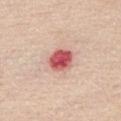<case>
  <biopsy_status>not biopsied; imaged during a skin examination</biopsy_status>
  <automated_metrics>
    <vs_skin_darker_L>18.0</vs_skin_darker_L>
    <vs_skin_contrast_norm>11.0</vs_skin_contrast_norm>
    <border_irregularity_0_10>1.5</border_irregularity_0_10>
    <color_variation_0_10>5.0</color_variation_0_10>
    <peripheral_color_asymmetry>1.5</peripheral_color_asymmetry>
  </automated_metrics>
  <lighting>white-light</lighting>
  <patient>
    <sex>male</sex>
    <age_approx>80</age_approx>
  </patient>
  <lesion_size>
    <long_diameter_mm_approx>3.0</long_diameter_mm_approx>
  </lesion_size>
  <site>chest</site>
  <image>
    <source>total-body photography crop</source>
    <field_of_view_mm>15</field_of_view_mm>
  </image>
</case>